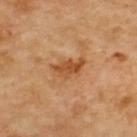Findings:
* biopsy status — no biopsy performed (imaged during a skin exam)
* anatomic site — the upper back
* lighting — cross-polarized
* image — total-body-photography crop, ~15 mm field of view
* diameter — ~4 mm (longest diameter)